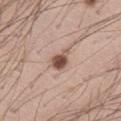Notes:
– biopsy status — total-body-photography surveillance lesion; no biopsy
– body site — the right thigh
– subject — male, aged around 40
– illumination — white-light illumination
– acquisition — ~15 mm tile from a whole-body skin photo
– diameter — ≈3 mm